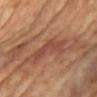subject — female, in their mid- to late 60s; site — the chest; image — ~15 mm crop, total-body skin-cancer survey.